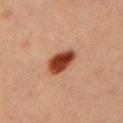No biopsy was performed on this lesion — it was imaged during a full skin examination and was not determined to be concerning. From the left upper arm. About 4 mm across. The total-body-photography lesion software estimated a lesion area of about 8 mm², an eccentricity of roughly 0.8, and two-axis asymmetry of about 0.2. It also reported an average lesion color of about L≈35 a*≈25 b*≈28 (CIELAB), a lesion–skin lightness drop of about 16, and a lesion-to-skin contrast of about 13.5 (normalized; higher = more distinct). It also reported a classifier nevus-likeness of about 100/100. A region of skin cropped from a whole-body photographic capture, roughly 15 mm wide. This is a cross-polarized tile. A male subject, in their 40s.